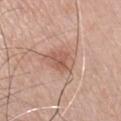The lesion was photographed on a routine skin check and not biopsied; there is no pathology result.
A 15 mm close-up extracted from a 3D total-body photography capture.
On the left upper arm.
Measured at roughly 4 mm in maximum diameter.
The subject is a male aged 73 to 77.